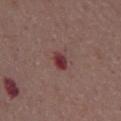{"biopsy_status": "not biopsied; imaged during a skin examination", "lesion_size": {"long_diameter_mm_approx": 2.5}, "image": {"source": "total-body photography crop", "field_of_view_mm": 15}, "patient": {"sex": "male", "age_approx": 70}, "site": "front of the torso"}